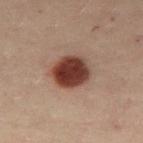Q: Was this lesion biopsied?
A: total-body-photography surveillance lesion; no biopsy
Q: What are the patient's age and sex?
A: female, approximately 30 years of age
Q: Illumination type?
A: cross-polarized illumination
Q: What kind of image is this?
A: ~15 mm crop, total-body skin-cancer survey
Q: What is the anatomic site?
A: the left leg